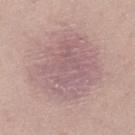automated lesion analysis — an area of roughly 45 mm² and an eccentricity of roughly 0.55; an average lesion color of about L≈60 a*≈19 b*≈18 (CIELAB), about 8 CIELAB-L* units darker than the surrounding skin, and a normalized lesion–skin contrast near 6; a color-variation rating of about 3.5/10 | subject — female, aged around 25 | image — total-body-photography crop, ~15 mm field of view | location — the leg.